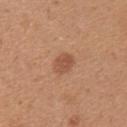No biopsy was performed on this lesion — it was imaged during a full skin examination and was not determined to be concerning.
An algorithmic analysis of the crop reported a lesion color around L≈52 a*≈23 b*≈33 in CIELAB, roughly 9 lightness units darker than nearby skin, and a normalized border contrast of about 6.5. The software also gave radial color variation of about 0.5.
A female subject aged around 25.
From the upper back.
A 15 mm close-up extracted from a 3D total-body photography capture.
The lesion's longest dimension is about 3 mm.